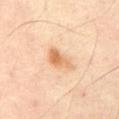{
  "biopsy_status": "not biopsied; imaged during a skin examination",
  "site": "abdomen",
  "lesion_size": {
    "long_diameter_mm_approx": 4.0
  },
  "image": {
    "source": "total-body photography crop",
    "field_of_view_mm": 15
  },
  "patient": {
    "sex": "male",
    "age_approx": 65
  },
  "lighting": "cross-polarized"
}